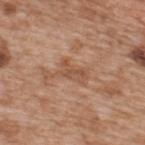Assessment: Captured during whole-body skin photography for melanoma surveillance; the lesion was not biopsied. Background: Imaged with white-light lighting. Automated tile analysis of the lesion measured a lesion area of about 5.5 mm², a shape eccentricity near 0.85, and a shape-asymmetry score of about 0.3 (0 = symmetric). On the upper back. The subject is a male aged 63 to 67. A 15 mm crop from a total-body photograph taken for skin-cancer surveillance.